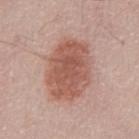Recorded during total-body skin imaging; not selected for excision or biopsy. A male subject aged around 50. From the front of the torso. This is a white-light tile. The lesion's longest dimension is about 7 mm. A 15 mm close-up tile from a total-body photography series done for melanoma screening. The lesion-visualizer software estimated an average lesion color of about L≈56 a*≈23 b*≈27 (CIELAB) and a normalized border contrast of about 8. The software also gave a nevus-likeness score of about 85/100 and a lesion-detection confidence of about 100/100.